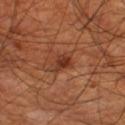biopsy status=total-body-photography surveillance lesion; no biopsy
illumination=cross-polarized
patient=male, about 70 years old
acquisition=~15 mm tile from a whole-body skin photo
site=the leg
size=≈2.5 mm